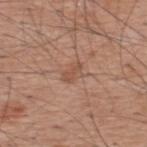– biopsy status · catalogued during a skin exam; not biopsied
– imaging modality · ~15 mm tile from a whole-body skin photo
– size · ≈2.5 mm
– site · the upper back
– patient · male, aged around 60
– automated metrics · an average lesion color of about L≈52 a*≈21 b*≈30 (CIELAB), about 7 CIELAB-L* units darker than the surrounding skin, and a normalized border contrast of about 5.5; border irregularity of about 3 on a 0–10 scale, internal color variation of about 0.5 on a 0–10 scale, and a peripheral color-asymmetry measure near 0
– tile lighting · white-light illumination